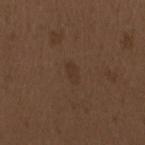Recorded during total-body skin imaging; not selected for excision or biopsy.